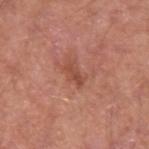biopsy_status: not biopsied; imaged during a skin examination
site: left forearm
automated_metrics:
  nevus_likeness_0_100: 5
  lesion_detection_confidence_0_100: 100
image:
  source: total-body photography crop
  field_of_view_mm: 15
lighting: white-light
patient:
  sex: male
  age_approx: 55